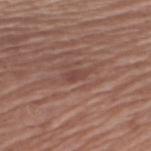Q: What did automated image analysis measure?
A: a lesion area of about 3 mm², a shape eccentricity near 0.9, and a shape-asymmetry score of about 0.2 (0 = symmetric); a nevus-likeness score of about 0/100
Q: Where on the body is the lesion?
A: the arm
Q: What lighting was used for the tile?
A: white-light illumination
Q: What kind of image is this?
A: total-body-photography crop, ~15 mm field of view
Q: What are the patient's age and sex?
A: female, aged around 80
Q: What is the lesion's diameter?
A: ~3 mm (longest diameter)